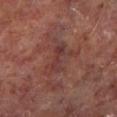| feature | finding |
|---|---|
| follow-up | total-body-photography surveillance lesion; no biopsy |